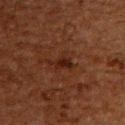No biopsy was performed on this lesion — it was imaged during a full skin examination and was not determined to be concerning.
Located on the back.
Automated image analysis of the tile measured an average lesion color of about L≈17 a*≈18 b*≈21 (CIELAB), a lesion–skin lightness drop of about 6, and a lesion-to-skin contrast of about 7.5 (normalized; higher = more distinct). And it measured a border-irregularity rating of about 5/10, a color-variation rating of about 1.5/10, and a peripheral color-asymmetry measure near 0.5.
A male patient, aged approximately 60.
Cropped from a whole-body photographic skin survey; the tile spans about 15 mm.